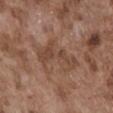Q: Was this lesion biopsied?
A: total-body-photography surveillance lesion; no biopsy
Q: What is the anatomic site?
A: the abdomen
Q: What are the patient's age and sex?
A: male, in their mid- to late 70s
Q: Lesion size?
A: about 6 mm
Q: How was the tile lit?
A: white-light illumination
Q: How was this image acquired?
A: total-body-photography crop, ~15 mm field of view